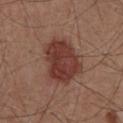Assessment:
This lesion was catalogued during total-body skin photography and was not selected for biopsy.
Clinical summary:
The lesion's longest dimension is about 5.5 mm. A male subject, roughly 55 years of age. On the front of the torso. A 15 mm close-up extracted from a 3D total-body photography capture. Automated image analysis of the tile measured a peripheral color-asymmetry measure near 1.5.